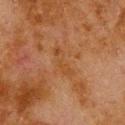follow-up — no biopsy performed (imaged during a skin exam)
lesion size — ~4 mm (longest diameter)
illumination — cross-polarized
subject — male, aged 78 to 82
anatomic site — the upper back
image source — total-body-photography crop, ~15 mm field of view